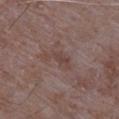No biopsy was performed on this lesion — it was imaged during a full skin examination and was not determined to be concerning. A male patient, approximately 65 years of age. The lesion's longest dimension is about 4 mm. A region of skin cropped from a whole-body photographic capture, roughly 15 mm wide. The tile uses white-light illumination. On the left upper arm.